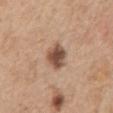Captured during whole-body skin photography for melanoma surveillance; the lesion was not biopsied. Approximately 3.5 mm at its widest. The tile uses white-light illumination. The lesion is on the mid back. A 15 mm crop from a total-body photograph taken for skin-cancer surveillance. A male subject roughly 65 years of age.